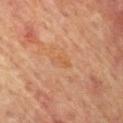Measured at roughly 3 mm in maximum diameter. A male subject, aged 63 to 67. Located on the mid back. Cropped from a total-body skin-imaging series; the visible field is about 15 mm. Automated image analysis of the tile measured a border-irregularity rating of about 3.5/10 and a peripheral color-asymmetry measure near 0.5. The software also gave lesion-presence confidence of about 100/100. This is a cross-polarized tile.